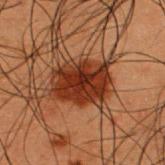| field | value |
|---|---|
| follow-up | catalogued during a skin exam; not biopsied |
| subject | male, aged 48–52 |
| anatomic site | the back |
| acquisition | ~15 mm crop, total-body skin-cancer survey |